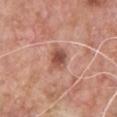Findings:
• follow-up — no biopsy performed (imaged during a skin exam)
• imaging modality — ~15 mm crop, total-body skin-cancer survey
• anatomic site — the front of the torso
• automated lesion analysis — an outline eccentricity of about 0.65 (0 = round, 1 = elongated) and a symmetry-axis asymmetry near 0.25
• patient — male, in their 60s